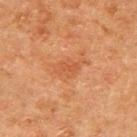biopsy_status: not biopsied; imaged during a skin examination
patient:
  sex: male
  age_approx: 65
automated_metrics:
  area_mm2_approx: 5.0
  eccentricity: 0.8
  cielab_L: 48
  cielab_a: 25
  cielab_b: 36
  vs_skin_darker_L: 6.0
  vs_skin_contrast_norm: 5.0
  border_irregularity_0_10: 3.5
  color_variation_0_10: 1.5
  peripheral_color_asymmetry: 0.5
  nevus_likeness_0_100: 0
image:
  source: total-body photography crop
  field_of_view_mm: 15
site: arm
lighting: cross-polarized
lesion_size:
  long_diameter_mm_approx: 3.5